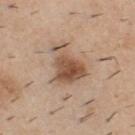Clinical impression:
The lesion was tiled from a total-body skin photograph and was not biopsied.
Context:
From the chest. A roughly 15 mm field-of-view crop from a total-body skin photograph. A male subject, roughly 40 years of age.